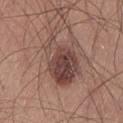Part of a total-body skin-imaging series; this lesion was reviewed on a skin check and was not flagged for biopsy. The tile uses white-light illumination. Located on the left thigh. A male patient aged around 25. Cropped from a total-body skin-imaging series; the visible field is about 15 mm.